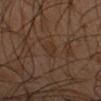The lesion was photographed on a routine skin check and not biopsied; there is no pathology result.
Automated tile analysis of the lesion measured an eccentricity of roughly 0.85 and two-axis asymmetry of about 0.3. The analysis additionally found a lesion–skin lightness drop of about 4. The software also gave a color-variation rating of about 1.5/10 and radial color variation of about 0.5. The software also gave an automated nevus-likeness rating near 0 out of 100 and a lesion-detection confidence of about 100/100.
A 15 mm close-up extracted from a 3D total-body photography capture.
Imaged with cross-polarized lighting.
The lesion is located on the left forearm.
A male patient, aged 48–52.